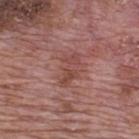Recorded during total-body skin imaging; not selected for excision or biopsy.
A region of skin cropped from a whole-body photographic capture, roughly 15 mm wide.
A male patient, aged 68–72.
The tile uses white-light illumination.
Located on the upper back.
About 4 mm across.